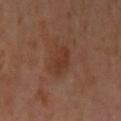biopsy status: catalogued during a skin exam; not biopsied